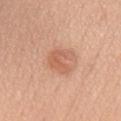• biopsy status · catalogued during a skin exam; not biopsied
• tile lighting · white-light
• body site · the chest
• patient · female, roughly 55 years of age
• image source · 15 mm crop, total-body photography
• diameter · ~3.5 mm (longest diameter)